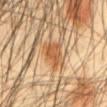Captured during whole-body skin photography for melanoma surveillance; the lesion was not biopsied.
The tile uses cross-polarized illumination.
A male subject aged approximately 45.
A lesion tile, about 15 mm wide, cut from a 3D total-body photograph.
Located on the abdomen.
Automated image analysis of the tile measured border irregularity of about 4 on a 0–10 scale and a within-lesion color-variation index near 3.5/10. The analysis additionally found a lesion-detection confidence of about 100/100.